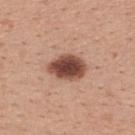biopsy status=imaged on a skin check; not biopsied
patient=male, aged 28 to 32
image source=~15 mm tile from a whole-body skin photo
lighting=white-light
site=the upper back
automated lesion analysis=an average lesion color of about L≈47 a*≈23 b*≈27 (CIELAB) and about 19 CIELAB-L* units darker than the surrounding skin
lesion diameter=about 4.5 mm